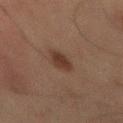biopsy status = no biopsy performed (imaged during a skin exam) | image source = ~15 mm tile from a whole-body skin photo | lesion diameter = ≈3.5 mm | anatomic site = the abdomen | patient = male, aged around 65 | tile lighting = cross-polarized illumination.